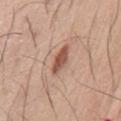Case summary:
• workup — total-body-photography surveillance lesion; no biopsy
• lighting — white-light illumination
• automated metrics — a lesion area of about 6 mm² and an outline eccentricity of about 0.9 (0 = round, 1 = elongated); a lesion color around L≈54 a*≈22 b*≈29 in CIELAB, a lesion–skin lightness drop of about 13, and a normalized lesion–skin contrast near 9
• acquisition — total-body-photography crop, ~15 mm field of view
• site — the chest
• patient — male, in their mid-70s
• diameter — ≈4 mm